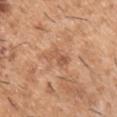Assessment:
Captured during whole-body skin photography for melanoma surveillance; the lesion was not biopsied.
Image and clinical context:
The total-body-photography lesion software estimated an average lesion color of about L≈56 a*≈23 b*≈34 (CIELAB), roughly 9 lightness units darker than nearby skin, and a lesion-to-skin contrast of about 6 (normalized; higher = more distinct). The analysis additionally found a color-variation rating of about 2.5/10 and a peripheral color-asymmetry measure near 1. The analysis additionally found a nevus-likeness score of about 0/100 and a detector confidence of about 100 out of 100 that the crop contains a lesion. Cropped from a total-body skin-imaging series; the visible field is about 15 mm. Located on the left upper arm. The patient is a male about 40 years old.